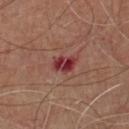Q: Was a biopsy performed?
A: catalogued during a skin exam; not biopsied
Q: Lesion location?
A: the chest
Q: How was the tile lit?
A: cross-polarized
Q: What is the imaging modality?
A: total-body-photography crop, ~15 mm field of view
Q: What did automated image analysis measure?
A: a footprint of about 5.5 mm² and a shape eccentricity near 0.75; a classifier nevus-likeness of about 0/100 and a detector confidence of about 100 out of 100 that the crop contains a lesion
Q: What are the patient's age and sex?
A: male, aged around 65